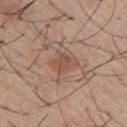| field | value |
|---|---|
| biopsy status | no biopsy performed (imaged during a skin exam) |
| tile lighting | white-light illumination |
| subject | male, aged 53 to 57 |
| diameter | ≈3.5 mm |
| automated metrics | a footprint of about 5.5 mm² and an outline eccentricity of about 0.75 (0 = round, 1 = elongated); a mean CIELAB color near L≈51 a*≈20 b*≈28, about 8 CIELAB-L* units darker than the surrounding skin, and a normalized border contrast of about 6; border irregularity of about 3.5 on a 0–10 scale; a nevus-likeness score of about 20/100 and a lesion-detection confidence of about 100/100 |
| image | ~15 mm crop, total-body skin-cancer survey |